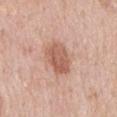<lesion>
  <biopsy_status>not biopsied; imaged during a skin examination</biopsy_status>
  <site>mid back</site>
  <patient>
    <sex>male</sex>
    <age_approx>60</age_approx>
  </patient>
  <lesion_size>
    <long_diameter_mm_approx>4.5</long_diameter_mm_approx>
  </lesion_size>
  <lighting>white-light</lighting>
  <image>
    <source>total-body photography crop</source>
    <field_of_view_mm>15</field_of_view_mm>
  </image>
</lesion>